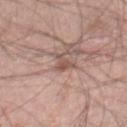notes = imaged on a skin check; not biopsied | image source = 15 mm crop, total-body photography | subject = male, approximately 60 years of age | location = the right lower leg | diameter = ~3 mm (longest diameter) | tile lighting = white-light illumination.